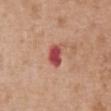No biopsy was performed on this lesion — it was imaged during a full skin examination and was not determined to be concerning.
Located on the chest.
Imaged with white-light lighting.
The recorded lesion diameter is about 3 mm.
Automated image analysis of the tile measured an average lesion color of about L≈49 a*≈34 b*≈26 (CIELAB), a lesion–skin lightness drop of about 15, and a normalized lesion–skin contrast near 10.5. And it measured a border-irregularity index near 2/10, a within-lesion color-variation index near 3.5/10, and a peripheral color-asymmetry measure near 1. The analysis additionally found a classifier nevus-likeness of about 0/100.
The patient is a female about 75 years old.
A 15 mm close-up extracted from a 3D total-body photography capture.